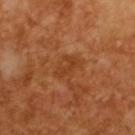Background:
A 15 mm close-up tile from a total-body photography series done for melanoma screening. The recorded lesion diameter is about 2.5 mm. A male subject, aged around 65.